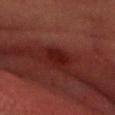Assessment: Part of a total-body skin-imaging series; this lesion was reviewed on a skin check and was not flagged for biopsy. Background: A male subject aged 58 to 62. This is a cross-polarized tile. Approximately 3 mm at its widest. A region of skin cropped from a whole-body photographic capture, roughly 15 mm wide. The lesion is located on the head or neck. The lesion-visualizer software estimated a lesion area of about 4.5 mm² and a shape eccentricity near 0.75. And it measured an average lesion color of about L≈15 a*≈23 b*≈18 (CIELAB), roughly 6 lightness units darker than nearby skin, and a lesion-to-skin contrast of about 8.5 (normalized; higher = more distinct). The analysis additionally found border irregularity of about 2 on a 0–10 scale and radial color variation of about 0.5.